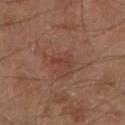Clinical impression:
Recorded during total-body skin imaging; not selected for excision or biopsy.
Image and clinical context:
The subject is a male aged 68 to 72. On the left lower leg. An algorithmic analysis of the crop reported an outline eccentricity of about 0.9 (0 = round, 1 = elongated) and a shape-asymmetry score of about 0.45 (0 = symmetric). And it measured a lesion color around L≈38 a*≈22 b*≈26 in CIELAB, about 6 CIELAB-L* units darker than the surrounding skin, and a normalized lesion–skin contrast near 5. And it measured a color-variation rating of about 0/10 and radial color variation of about 0. The analysis additionally found an automated nevus-likeness rating near 15 out of 100. This is a cross-polarized tile. A 15 mm crop from a total-body photograph taken for skin-cancer surveillance.